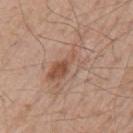follow-up: no biopsy performed (imaged during a skin exam)
location: the left upper arm
image: 15 mm crop, total-body photography
subject: male, aged 68 to 72